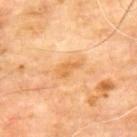The lesion was tiled from a total-body skin photograph and was not biopsied. Captured under cross-polarized illumination. The recorded lesion diameter is about 3.5 mm. The lesion is on the upper back. Cropped from a total-body skin-imaging series; the visible field is about 15 mm. A male subject about 65 years old. The lesion-visualizer software estimated a lesion-to-skin contrast of about 6 (normalized; higher = more distinct). The software also gave a color-variation rating of about 1.5/10 and peripheral color asymmetry of about 0.5.